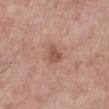Imaged during a routine full-body skin examination; the lesion was not biopsied and no histopathology is available. A female patient, in their 70s. Captured under white-light illumination. Cropped from a total-body skin-imaging series; the visible field is about 15 mm. On the right lower leg. The recorded lesion diameter is about 3 mm. Automated image analysis of the tile measured a lesion area of about 4.5 mm² and a shape-asymmetry score of about 0.3 (0 = symmetric). The analysis additionally found a mean CIELAB color near L≈53 a*≈23 b*≈29, a lesion–skin lightness drop of about 10, and a normalized border contrast of about 7.